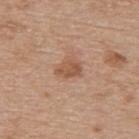- workup · imaged on a skin check; not biopsied
- lesion diameter · ≈3 mm
- subject · female, roughly 65 years of age
- location · the upper back
- acquisition · total-body-photography crop, ~15 mm field of view